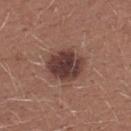Part of a total-body skin-imaging series; this lesion was reviewed on a skin check and was not flagged for biopsy.